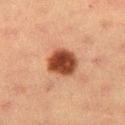The lesion was tiled from a total-body skin photograph and was not biopsied. The subject is a female roughly 55 years of age. The lesion's longest dimension is about 4 mm. This is a cross-polarized tile. Located on the left thigh. Automated image analysis of the tile measured an area of roughly 11 mm² and an outline eccentricity of about 0.5 (0 = round, 1 = elongated). And it measured an average lesion color of about L≈37 a*≈23 b*≈30 (CIELAB), about 16 CIELAB-L* units darker than the surrounding skin, and a lesion-to-skin contrast of about 13 (normalized; higher = more distinct). It also reported an automated nevus-likeness rating near 100 out of 100 and a detector confidence of about 100 out of 100 that the crop contains a lesion. A close-up tile cropped from a whole-body skin photograph, about 15 mm across.